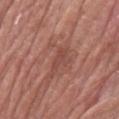Recorded during total-body skin imaging; not selected for excision or biopsy. This is a white-light tile. A region of skin cropped from a whole-body photographic capture, roughly 15 mm wide. From the left forearm. A male subject aged 78 to 82. Approximately 3.5 mm at its widest.